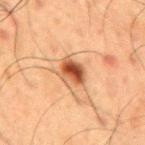Captured during whole-body skin photography for melanoma surveillance; the lesion was not biopsied.
The lesion's longest dimension is about 3.5 mm.
A close-up tile cropped from a whole-body skin photograph, about 15 mm across.
The patient is a male roughly 60 years of age.
The lesion is located on the back.
Automated image analysis of the tile measured a lesion area of about 7 mm², an outline eccentricity of about 0.65 (0 = round, 1 = elongated), and two-axis asymmetry of about 0.25.